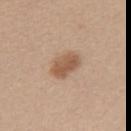  site: back
  automated_metrics:
    cielab_L: 55
    cielab_a: 19
    cielab_b: 31
    vs_skin_darker_L: 11.0
    vs_skin_contrast_norm: 8.0
    nevus_likeness_0_100: 90
    lesion_detection_confidence_0_100: 100
  image:
    source: total-body photography crop
    field_of_view_mm: 15
  lesion_size:
    long_diameter_mm_approx: 3.5
  patient:
    sex: female
    age_approx: 40